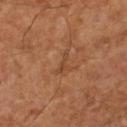This lesion was catalogued during total-body skin photography and was not selected for biopsy. Automated tile analysis of the lesion measured a lesion area of about 2.5 mm², an outline eccentricity of about 0.9 (0 = round, 1 = elongated), and a symmetry-axis asymmetry near 0.6. It also reported a lesion color around L≈40 a*≈21 b*≈31 in CIELAB and about 6 CIELAB-L* units darker than the surrounding skin. And it measured a color-variation rating of about 0/10 and a peripheral color-asymmetry measure near 0. And it measured an automated nevus-likeness rating near 0 out of 100 and lesion-presence confidence of about 100/100. Longest diameter approximately 3 mm. Cropped from a whole-body photographic skin survey; the tile spans about 15 mm. The tile uses cross-polarized illumination. From the right lower leg. A male patient in their 60s.